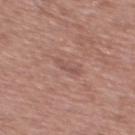Clinical impression:
Part of a total-body skin-imaging series; this lesion was reviewed on a skin check and was not flagged for biopsy.
Acquisition and patient details:
Approximately 3 mm at its widest. Imaged with white-light lighting. A male patient, aged 43–47. A 15 mm crop from a total-body photograph taken for skin-cancer surveillance. Automated image analysis of the tile measured an area of roughly 2.5 mm², an eccentricity of roughly 0.95, and a symmetry-axis asymmetry near 0.4. And it measured a normalized lesion–skin contrast near 5. And it measured an automated nevus-likeness rating near 0 out of 100 and a lesion-detection confidence of about 100/100. The lesion is on the chest.